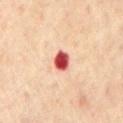Imaged with cross-polarized lighting. This image is a 15 mm lesion crop taken from a total-body photograph. The patient is a male in their mid- to late 60s. The lesion is located on the chest. Automated tile analysis of the lesion measured a border-irregularity rating of about 2/10, internal color variation of about 4 on a 0–10 scale, and radial color variation of about 1.